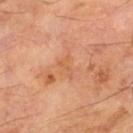The lesion was photographed on a routine skin check and not biopsied; there is no pathology result.
Imaged with cross-polarized lighting.
A region of skin cropped from a whole-body photographic capture, roughly 15 mm wide.
About 2.5 mm across.
On the left thigh.
A male subject, aged approximately 70.